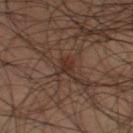Impression: The lesion was photographed on a routine skin check and not biopsied; there is no pathology result. Acquisition and patient details: Longest diameter approximately 3 mm. The subject is a male about 40 years old. The total-body-photography lesion software estimated a mean CIELAB color near L≈30 a*≈17 b*≈23, roughly 7 lightness units darker than nearby skin, and a normalized lesion–skin contrast near 7. The analysis additionally found a nevus-likeness score of about 0/100 and a detector confidence of about 75 out of 100 that the crop contains a lesion. A lesion tile, about 15 mm wide, cut from a 3D total-body photograph. The lesion is on the left lower leg. This is a cross-polarized tile.